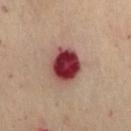Q: Is there a histopathology result?
A: imaged on a skin check; not biopsied
Q: What are the patient's age and sex?
A: female, aged 58–62
Q: Lesion location?
A: the lower back
Q: What kind of image is this?
A: ~15 mm crop, total-body skin-cancer survey
Q: Lesion size?
A: about 5 mm
Q: Automated lesion metrics?
A: a footprint of about 14 mm², an eccentricity of roughly 0.65, and a shape-asymmetry score of about 0.1 (0 = symmetric); a lesion color around L≈40 a*≈30 b*≈22 in CIELAB, a lesion–skin lightness drop of about 22, and a normalized border contrast of about 15.5